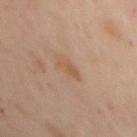| key | value |
|---|---|
| workup | catalogued during a skin exam; not biopsied |
| lesion size | ~2.5 mm (longest diameter) |
| image source | ~15 mm crop, total-body skin-cancer survey |
| patient | female, aged around 55 |
| lighting | cross-polarized |
| automated metrics | a lesion area of about 2.5 mm², an outline eccentricity of about 0.9 (0 = round, 1 = elongated), and two-axis asymmetry of about 0.4; an average lesion color of about L≈45 a*≈16 b*≈27 (CIELAB), about 6 CIELAB-L* units darker than the surrounding skin, and a lesion-to-skin contrast of about 5.5 (normalized; higher = more distinct); a classifier nevus-likeness of about 5/100 |
| location | the chest |